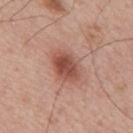Impression:
This lesion was catalogued during total-body skin photography and was not selected for biopsy.
Acquisition and patient details:
This is a white-light tile. A roughly 15 mm field-of-view crop from a total-body skin photograph. Measured at roughly 4 mm in maximum diameter. The lesion-visualizer software estimated a footprint of about 8.5 mm², an eccentricity of roughly 0.75, and two-axis asymmetry of about 0.15. The analysis additionally found a lesion color around L≈50 a*≈25 b*≈28 in CIELAB. It also reported a classifier nevus-likeness of about 95/100 and lesion-presence confidence of about 100/100. The lesion is on the mid back. The patient is a male in their mid- to late 60s.